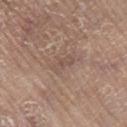Captured during whole-body skin photography for melanoma surveillance; the lesion was not biopsied.
The total-body-photography lesion software estimated a footprint of about 3 mm², a shape eccentricity near 0.9, and a symmetry-axis asymmetry near 0.35. It also reported roughly 6 lightness units darker than nearby skin. And it measured border irregularity of about 4 on a 0–10 scale, a color-variation rating of about 0/10, and peripheral color asymmetry of about 0. The software also gave a nevus-likeness score of about 0/100 and a lesion-detection confidence of about 70/100.
A roughly 15 mm field-of-view crop from a total-body skin photograph.
A female patient approximately 75 years of age.
The recorded lesion diameter is about 3 mm.
Captured under white-light illumination.
The lesion is located on the right lower leg.